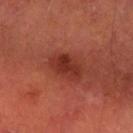workup: no biopsy performed (imaged during a skin exam); imaging modality: ~15 mm crop, total-body skin-cancer survey; site: the right forearm; subject: male, in their mid-60s.